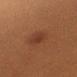{"image": {"source": "total-body photography crop", "field_of_view_mm": 15}, "lesion_size": {"long_diameter_mm_approx": 2.5}, "patient": {"sex": "female", "age_approx": 55}, "lighting": "cross-polarized", "automated_metrics": {"eccentricity": 0.7, "shape_asymmetry": 0.3, "cielab_L": 28, "cielab_a": 20, "cielab_b": 25, "vs_skin_darker_L": 5.0, "vs_skin_contrast_norm": 5.5, "border_irregularity_0_10": 2.5, "color_variation_0_10": 1.5, "peripheral_color_asymmetry": 0.5}, "site": "left upper arm"}